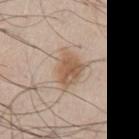<record>
<biopsy_status>not biopsied; imaged during a skin examination</biopsy_status>
<image>
  <source>total-body photography crop</source>
  <field_of_view_mm>15</field_of_view_mm>
</image>
<lighting>white-light</lighting>
<patient>
  <sex>male</sex>
  <age_approx>65</age_approx>
</patient>
<lesion_size>
  <long_diameter_mm_approx>5.0</long_diameter_mm_approx>
</lesion_size>
<automated_metrics>
  <area_mm2_approx>11.0</area_mm2_approx>
  <shape_asymmetry>0.35</shape_asymmetry>
</automated_metrics>
<site>front of the torso</site>
</record>